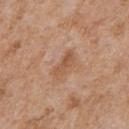Recorded during total-body skin imaging; not selected for excision or biopsy.
Imaged with white-light lighting.
An algorithmic analysis of the crop reported a lesion area of about 4.5 mm², an outline eccentricity of about 0.9 (0 = round, 1 = elongated), and a symmetry-axis asymmetry near 0.3. It also reported about 8 CIELAB-L* units darker than the surrounding skin. The software also gave a within-lesion color-variation index near 2.5/10 and radial color variation of about 1. The analysis additionally found a classifier nevus-likeness of about 0/100 and lesion-presence confidence of about 100/100.
About 3.5 mm across.
The lesion is located on the chest.
A male subject, aged around 65.
This image is a 15 mm lesion crop taken from a total-body photograph.